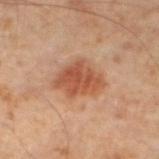No biopsy was performed on this lesion — it was imaged during a full skin examination and was not determined to be concerning.
The patient is a male aged 58 to 62.
A 15 mm crop from a total-body photograph taken for skin-cancer surveillance.
Located on the left lower leg.
The total-body-photography lesion software estimated an area of roughly 14 mm² and a shape eccentricity near 0.7. The analysis additionally found a border-irregularity index near 2.5/10, internal color variation of about 3.5 on a 0–10 scale, and a peripheral color-asymmetry measure near 1. And it measured a classifier nevus-likeness of about 90/100 and a detector confidence of about 100 out of 100 that the crop contains a lesion.
Captured under cross-polarized illumination.